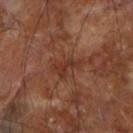Assessment:
Recorded during total-body skin imaging; not selected for excision or biopsy.
Image and clinical context:
A male subject in their mid- to late 60s. The lesion is located on the right forearm. An algorithmic analysis of the crop reported a classifier nevus-likeness of about 0/100 and lesion-presence confidence of about 90/100. Cropped from a whole-body photographic skin survey; the tile spans about 15 mm. The recorded lesion diameter is about 3.5 mm. Imaged with cross-polarized lighting.